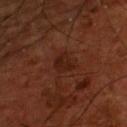<tbp_lesion>
<biopsy_status>not biopsied; imaged during a skin examination</biopsy_status>
</tbp_lesion>